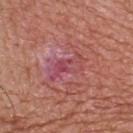  biopsy_status: not biopsied; imaged during a skin examination
  image:
    source: total-body photography crop
    field_of_view_mm: 15
  automated_metrics:
    shape_asymmetry: 0.4
    cielab_L: 48
    cielab_a: 32
    cielab_b: 20
    vs_skin_contrast_norm: 6.0
  lighting: white-light
  site: upper back
  patient:
    sex: male
    age_approx: 65
  lesion_size:
    long_diameter_mm_approx: 5.0